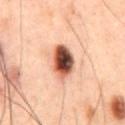Part of a total-body skin-imaging series; this lesion was reviewed on a skin check and was not flagged for biopsy. A 15 mm close-up extracted from a 3D total-body photography capture. The tile uses cross-polarized illumination. A male patient, about 60 years old. Located on the front of the torso.